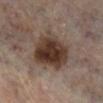| feature | finding |
|---|---|
| workup | imaged on a skin check; not biopsied |